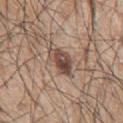Q: Was this lesion biopsied?
A: no biopsy performed (imaged during a skin exam)
Q: Automated lesion metrics?
A: a mean CIELAB color near L≈47 a*≈17 b*≈24, about 13 CIELAB-L* units darker than the surrounding skin, and a normalized lesion–skin contrast near 9.5
Q: Illumination type?
A: white-light
Q: What is the anatomic site?
A: the left arm
Q: What is the imaging modality?
A: ~15 mm crop, total-body skin-cancer survey
Q: Who is the patient?
A: male, about 70 years old
Q: What is the lesion's diameter?
A: ~4.5 mm (longest diameter)